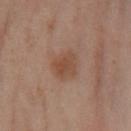notes: catalogued during a skin exam; not biopsied | patient: female, about 55 years old | anatomic site: the left lower leg | diameter: about 3 mm | image source: ~15 mm tile from a whole-body skin photo.